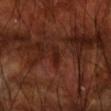biopsy status = no biopsy performed (imaged during a skin exam) | site = the arm | lighting = cross-polarized | lesion size = ≈2.5 mm | TBP lesion metrics = a lesion color around L≈21 a*≈23 b*≈25 in CIELAB and roughly 5 lightness units darker than nearby skin; an automated nevus-likeness rating near 0 out of 100 and lesion-presence confidence of about 55/100 | subject = male, aged approximately 70 | imaging modality = ~15 mm tile from a whole-body skin photo.